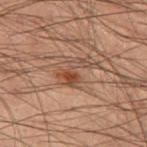This lesion was catalogued during total-body skin photography and was not selected for biopsy.
A male patient in their mid-50s.
On the left thigh.
Captured under cross-polarized illumination.
A 15 mm close-up extracted from a 3D total-body photography capture.